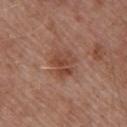biopsy status=imaged on a skin check; not biopsied | imaging modality=total-body-photography crop, ~15 mm field of view | size=~3.5 mm (longest diameter) | body site=the upper back | subject=male, in their mid- to late 50s | automated metrics=an average lesion color of about L≈45 a*≈23 b*≈29 (CIELAB) and a normalized border contrast of about 7; an automated nevus-likeness rating near 40 out of 100 and lesion-presence confidence of about 100/100 | tile lighting=white-light illumination.